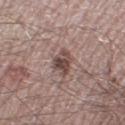Clinical impression: The lesion was tiled from a total-body skin photograph and was not biopsied. Image and clinical context: This is a white-light tile. A 15 mm crop from a total-body photograph taken for skin-cancer surveillance. The patient is a male aged around 50. On the right thigh. Automated tile analysis of the lesion measured a footprint of about 6 mm² and an outline eccentricity of about 0.75 (0 = round, 1 = elongated). The software also gave a border-irregularity index near 2.5/10, a color-variation rating of about 5/10, and radial color variation of about 2. It also reported a classifier nevus-likeness of about 65/100. About 3 mm across.